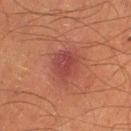Q: Illumination type?
A: cross-polarized illumination
Q: What did automated image analysis measure?
A: an eccentricity of roughly 0.6 and two-axis asymmetry of about 0.2; a mean CIELAB color near L≈35 a*≈25 b*≈22 and roughly 7 lightness units darker than nearby skin; a border-irregularity index near 2.5/10 and a color-variation rating of about 3/10
Q: Lesion location?
A: the left lower leg
Q: What is the lesion's diameter?
A: ≈4 mm
Q: How was this image acquired?
A: ~15 mm crop, total-body skin-cancer survey
Q: Patient demographics?
A: male, about 65 years old